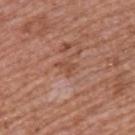workup=no biopsy performed (imaged during a skin exam)
lesion size=about 2.5 mm
image source=15 mm crop, total-body photography
illumination=white-light
automated lesion analysis=a lesion color around L≈49 a*≈23 b*≈31 in CIELAB, about 7 CIELAB-L* units darker than the surrounding skin, and a normalized lesion–skin contrast near 6; a color-variation rating of about 0.5/10 and a peripheral color-asymmetry measure near 0
subject=male, in their mid-50s
body site=the upper back